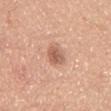Impression:
This lesion was catalogued during total-body skin photography and was not selected for biopsy.
Context:
On the lower back. A 15 mm close-up tile from a total-body photography series done for melanoma screening. Captured under white-light illumination. A male patient aged 48 to 52.